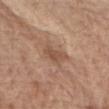Q: Was this lesion biopsied?
A: catalogued during a skin exam; not biopsied